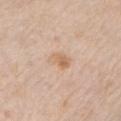Impression: Imaged during a routine full-body skin examination; the lesion was not biopsied and no histopathology is available. Context: The patient is a male aged 73–77. Captured under white-light illumination. The lesion's longest dimension is about 2.5 mm. The lesion is located on the left upper arm. A region of skin cropped from a whole-body photographic capture, roughly 15 mm wide. An algorithmic analysis of the crop reported a lesion color around L≈65 a*≈18 b*≈33 in CIELAB, roughly 8 lightness units darker than nearby skin, and a lesion-to-skin contrast of about 6 (normalized; higher = more distinct). It also reported a border-irregularity index near 2.5/10 and peripheral color asymmetry of about 1.5. And it measured a classifier nevus-likeness of about 15/100 and lesion-presence confidence of about 100/100.